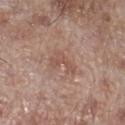Assessment:
This lesion was catalogued during total-body skin photography and was not selected for biopsy.
Clinical summary:
This image is a 15 mm lesion crop taken from a total-body photograph. Imaged with white-light lighting. A male patient, about 70 years old. The lesion is located on the right lower leg. The lesion's longest dimension is about 3.5 mm.